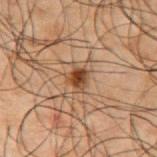Imaged during a routine full-body skin examination; the lesion was not biopsied and no histopathology is available. A male patient in their 50s. From the right upper arm. About 2.5 mm across. A 15 mm crop from a total-body photograph taken for skin-cancer surveillance.